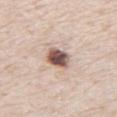{"biopsy_status": "not biopsied; imaged during a skin examination", "lighting": "white-light", "patient": {"sex": "male", "age_approx": 80}, "image": {"source": "total-body photography crop", "field_of_view_mm": 15}, "lesion_size": {"long_diameter_mm_approx": 3.0}, "site": "abdomen"}